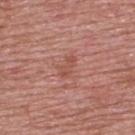follow-up = catalogued during a skin exam; not biopsied | image = total-body-photography crop, ~15 mm field of view | patient = male, about 50 years old | lighting = white-light | site = the upper back | size = about 3 mm.